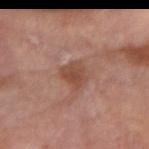Notes:
* follow-up — total-body-photography surveillance lesion; no biopsy
* body site — the right forearm
* image source — ~15 mm crop, total-body skin-cancer survey
* automated metrics — an eccentricity of roughly 0.55; border irregularity of about 3 on a 0–10 scale, internal color variation of about 2.5 on a 0–10 scale, and radial color variation of about 0.5; an automated nevus-likeness rating near 0 out of 100 and lesion-presence confidence of about 100/100
* lighting — cross-polarized illumination
* patient — male, in their mid-60s